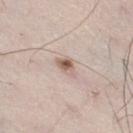<record>
<biopsy_status>not biopsied; imaged during a skin examination</biopsy_status>
<image>
  <source>total-body photography crop</source>
  <field_of_view_mm>15</field_of_view_mm>
</image>
<site>leg</site>
<patient>
  <sex>male</sex>
  <age_approx>35</age_approx>
</patient>
<lighting>white-light</lighting>
</record>